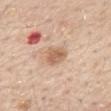Impression: Imaged during a routine full-body skin examination; the lesion was not biopsied and no histopathology is available. Context: The lesion is located on the mid back. A male patient, roughly 55 years of age. A close-up tile cropped from a whole-body skin photograph, about 15 mm across.